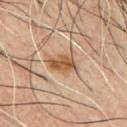Recorded during total-body skin imaging; not selected for excision or biopsy. A region of skin cropped from a whole-body photographic capture, roughly 15 mm wide. A male subject aged 48–52. From the chest. The recorded lesion diameter is about 3.5 mm.